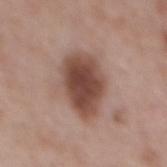Part of a total-body skin-imaging series; this lesion was reviewed on a skin check and was not flagged for biopsy. The lesion is on the mid back. A 15 mm crop from a total-body photograph taken for skin-cancer surveillance. Longest diameter approximately 6.5 mm. A male subject aged around 55. Captured under white-light illumination.